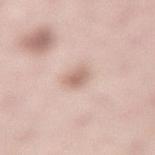No biopsy was performed on this lesion — it was imaged during a full skin examination and was not determined to be concerning. From the lower back. A 15 mm close-up tile from a total-body photography series done for melanoma screening. A female patient, roughly 50 years of age. The recorded lesion diameter is about 3 mm.